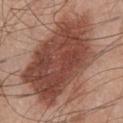{
  "image": {
    "source": "total-body photography crop",
    "field_of_view_mm": 15
  },
  "site": "chest",
  "patient": {
    "sex": "male",
    "age_approx": 45
  }
}